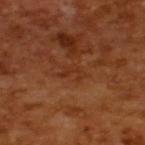The patient is a male in their mid-60s.
A 15 mm crop from a total-body photograph taken for skin-cancer surveillance.